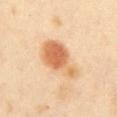biopsy_status: not biopsied; imaged during a skin examination
lesion_size:
  long_diameter_mm_approx: 6.0
image:
  source: total-body photography crop
  field_of_view_mm: 15
patient:
  sex: female
  age_approx: 60
site: abdomen
lighting: cross-polarized